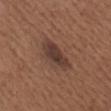workup: catalogued during a skin exam; not biopsied | lesion diameter: ≈4.5 mm | image source: ~15 mm crop, total-body skin-cancer survey | tile lighting: white-light illumination | body site: the chest | automated lesion analysis: a lesion color around L≈38 a*≈17 b*≈24 in CIELAB, about 9 CIELAB-L* units darker than the surrounding skin, and a normalized lesion–skin contrast near 8.5; border irregularity of about 3 on a 0–10 scale, a within-lesion color-variation index near 3.5/10, and peripheral color asymmetry of about 1; a nevus-likeness score of about 75/100 and lesion-presence confidence of about 100/100 | patient: male, in their mid-60s.